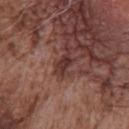Q: Is there a histopathology result?
A: no biopsy performed (imaged during a skin exam)
Q: What is the anatomic site?
A: the chest
Q: Automated lesion metrics?
A: an outline eccentricity of about 0.8 (0 = round, 1 = elongated) and two-axis asymmetry of about 0.25; an automated nevus-likeness rating near 5 out of 100 and a detector confidence of about 95 out of 100 that the crop contains a lesion
Q: What is the imaging modality?
A: 15 mm crop, total-body photography
Q: Who is the patient?
A: male, in their mid- to late 70s
Q: What lighting was used for the tile?
A: white-light